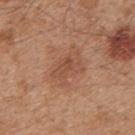A male patient, aged approximately 45.
About 4.5 mm across.
Captured under white-light illumination.
The lesion is on the upper back.
Cropped from a whole-body photographic skin survey; the tile spans about 15 mm.